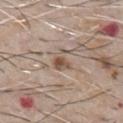Case summary:
– notes — catalogued during a skin exam; not biopsied
– anatomic site — the chest
– illumination — white-light illumination
– image source — total-body-photography crop, ~15 mm field of view
– size — ≈3 mm
– patient — male, aged around 65
– image-analysis metrics — a lesion color around L≈53 a*≈15 b*≈26 in CIELAB, about 10 CIELAB-L* units darker than the surrounding skin, and a normalized border contrast of about 7